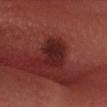Findings:
* workup — catalogued during a skin exam; not biopsied
* size — ≈4 mm
* acquisition — total-body-photography crop, ~15 mm field of view
* illumination — white-light illumination
* subject — male, aged 43 to 47
* body site — the head or neck